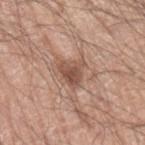{"biopsy_status": "not biopsied; imaged during a skin examination", "patient": {"sex": "male", "age_approx": 70}, "site": "left upper arm", "image": {"source": "total-body photography crop", "field_of_view_mm": 15}, "lighting": "white-light"}